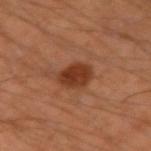Clinical impression:
No biopsy was performed on this lesion — it was imaged during a full skin examination and was not determined to be concerning.
Context:
Cropped from a whole-body photographic skin survey; the tile spans about 15 mm. Captured under cross-polarized illumination. From the right upper arm. The lesion's longest dimension is about 3.5 mm. A male patient, about 45 years old.